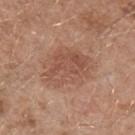Notes:
– workup · total-body-photography surveillance lesion; no biopsy
– lesion size · ≈5.5 mm
– location · the left upper arm
– TBP lesion metrics · an area of roughly 20 mm², an eccentricity of roughly 0.6, and two-axis asymmetry of about 0.2; about 8 CIELAB-L* units darker than the surrounding skin and a normalized border contrast of about 6; a border-irregularity rating of about 2.5/10, a within-lesion color-variation index near 3.5/10, and a peripheral color-asymmetry measure near 1.5; lesion-presence confidence of about 100/100
– image · ~15 mm crop, total-body skin-cancer survey
– patient · male, in their mid- to late 50s